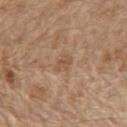Acquisition and patient details: A 15 mm crop from a total-body photograph taken for skin-cancer surveillance. About 2.5 mm across. This is a white-light tile. A male patient aged 68 to 72. The lesion is located on the chest.